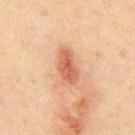The lesion was photographed on a routine skin check and not biopsied; there is no pathology result. The patient is a male roughly 50 years of age. From the abdomen. A region of skin cropped from a whole-body photographic capture, roughly 15 mm wide.